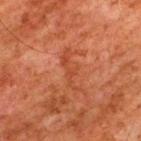The lesion was photographed on a routine skin check and not biopsied; there is no pathology result.
An algorithmic analysis of the crop reported an area of roughly 6 mm² and two-axis asymmetry of about 0.55. And it measured a lesion color around L≈37 a*≈26 b*≈31 in CIELAB, roughly 5 lightness units darker than nearby skin, and a lesion-to-skin contrast of about 5 (normalized; higher = more distinct). It also reported lesion-presence confidence of about 95/100.
A male subject, approximately 80 years of age.
This is a cross-polarized tile.
Located on the upper back.
A close-up tile cropped from a whole-body skin photograph, about 15 mm across.